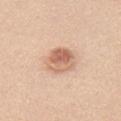Q: Was a biopsy performed?
A: imaged on a skin check; not biopsied
Q: Illumination type?
A: white-light illumination
Q: What is the anatomic site?
A: the chest
Q: Patient demographics?
A: female, aged around 40
Q: Automated lesion metrics?
A: an area of roughly 10 mm², an eccentricity of roughly 0.5, and two-axis asymmetry of about 0.15; an automated nevus-likeness rating near 85 out of 100
Q: What kind of image is this?
A: ~15 mm crop, total-body skin-cancer survey
Q: Lesion size?
A: about 4 mm